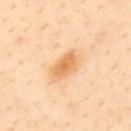Recorded during total-body skin imaging; not selected for excision or biopsy. A male patient, approximately 55 years of age. Longest diameter approximately 4 mm. Automated image analysis of the tile measured a shape eccentricity near 0.85 and two-axis asymmetry of about 0.2. It also reported a border-irregularity rating of about 2/10, a within-lesion color-variation index near 3/10, and a peripheral color-asymmetry measure near 0.5. Located on the upper back. The tile uses cross-polarized illumination. A 15 mm close-up tile from a total-body photography series done for melanoma screening.